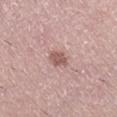* workup — no biopsy performed (imaged during a skin exam)
* patient — female, aged 38 to 42
* body site — the leg
* acquisition — total-body-photography crop, ~15 mm field of view